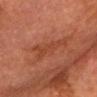Impression: No biopsy was performed on this lesion — it was imaged during a full skin examination and was not determined to be concerning. Acquisition and patient details: A male patient, aged around 75. Imaged with cross-polarized lighting. From the front of the torso. The recorded lesion diameter is about 5 mm. Cropped from a total-body skin-imaging series; the visible field is about 15 mm.